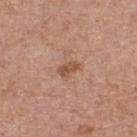notes: catalogued during a skin exam; not biopsied | lesion size: about 2.5 mm | image-analysis metrics: a footprint of about 3 mm², an outline eccentricity of about 0.85 (0 = round, 1 = elongated), and a shape-asymmetry score of about 0.25 (0 = symmetric); about 10 CIELAB-L* units darker than the surrounding skin and a lesion-to-skin contrast of about 7 (normalized; higher = more distinct); a border-irregularity rating of about 2.5/10, internal color variation of about 3 on a 0–10 scale, and radial color variation of about 1 | patient: male, aged 78–82 | image: total-body-photography crop, ~15 mm field of view | site: the upper back.